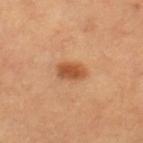Recorded during total-body skin imaging; not selected for excision or biopsy.
The lesion-visualizer software estimated a shape eccentricity near 0.8 and a symmetry-axis asymmetry near 0.2. The software also gave a border-irregularity index near 1.5/10 and a color-variation rating of about 4/10. The software also gave a classifier nevus-likeness of about 95/100 and a lesion-detection confidence of about 100/100.
Captured under cross-polarized illumination.
A region of skin cropped from a whole-body photographic capture, roughly 15 mm wide.
The lesion is located on the leg.
The recorded lesion diameter is about 3.5 mm.
A female subject in their 60s.